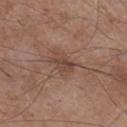Q: Is there a histopathology result?
A: no biopsy performed (imaged during a skin exam)
Q: How was the tile lit?
A: white-light
Q: What is the anatomic site?
A: the right lower leg
Q: Who is the patient?
A: male, approximately 55 years of age
Q: What is the imaging modality?
A: ~15 mm tile from a whole-body skin photo
Q: What is the lesion's diameter?
A: ~3 mm (longest diameter)